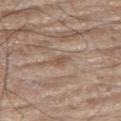<case>
<biopsy_status>not biopsied; imaged during a skin examination</biopsy_status>
<patient>
  <sex>male</sex>
  <age_approx>85+</age_approx>
</patient>
<lighting>white-light</lighting>
<automated_metrics>
  <area_mm2_approx>2.5</area_mm2_approx>
  <eccentricity>0.85</eccentricity>
  <shape_asymmetry>0.25</shape_asymmetry>
  <cielab_L>53</cielab_L>
  <cielab_a>16</cielab_a>
  <cielab_b>28</cielab_b>
  <vs_skin_darker_L>8.0</vs_skin_darker_L>
  <border_irregularity_0_10>2.5</border_irregularity_0_10>
  <color_variation_0_10>1.5</color_variation_0_10>
  <peripheral_color_asymmetry>0.5</peripheral_color_asymmetry>
  <nevus_likeness_0_100>0</nevus_likeness_0_100>
  <lesion_detection_confidence_0_100>80</lesion_detection_confidence_0_100>
</automated_metrics>
<image>
  <source>total-body photography crop</source>
  <field_of_view_mm>15</field_of_view_mm>
</image>
<site>left upper arm</site>
<lesion_size>
  <long_diameter_mm_approx>2.5</long_diameter_mm_approx>
</lesion_size>
</case>